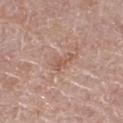workup = total-body-photography surveillance lesion; no biopsy | lighting = white-light illumination | site = the right thigh | subject = female, aged around 65 | diameter = about 3 mm | acquisition = total-body-photography crop, ~15 mm field of view.